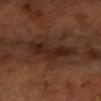Q: Is there a histopathology result?
A: total-body-photography surveillance lesion; no biopsy
Q: How was the tile lit?
A: cross-polarized
Q: What is the anatomic site?
A: the left forearm
Q: Who is the patient?
A: female, aged approximately 70
Q: What is the imaging modality?
A: ~15 mm tile from a whole-body skin photo